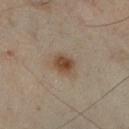{"biopsy_status": "not biopsied; imaged during a skin examination", "lighting": "cross-polarized", "image": {"source": "total-body photography crop", "field_of_view_mm": 15}, "patient": {"sex": "male", "age_approx": 55}, "lesion_size": {"long_diameter_mm_approx": 2.5}, "site": "right lower leg"}